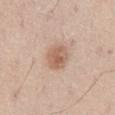workup = catalogued during a skin exam; not biopsied | image = ~15 mm crop, total-body skin-cancer survey | location = the right thigh | size = about 3 mm | subject = male, aged approximately 75 | illumination = white-light.